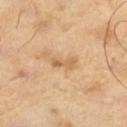Imaged during a routine full-body skin examination; the lesion was not biopsied and no histopathology is available.
A lesion tile, about 15 mm wide, cut from a 3D total-body photograph.
This is a cross-polarized tile.
A male subject, approximately 65 years of age.
The lesion's longest dimension is about 3 mm.
Automated image analysis of the tile measured border irregularity of about 4 on a 0–10 scale and peripheral color asymmetry of about 0. It also reported a classifier nevus-likeness of about 0/100.